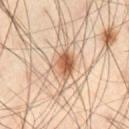Imaged during a routine full-body skin examination; the lesion was not biopsied and no histopathology is available.
About 3 mm across.
A male subject, roughly 55 years of age.
From the left thigh.
An algorithmic analysis of the crop reported a lesion area of about 7 mm², an eccentricity of roughly 0.4, and two-axis asymmetry of about 0.25.
A 15 mm close-up extracted from a 3D total-body photography capture.
The tile uses cross-polarized illumination.